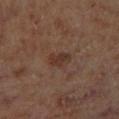Findings:
– location — the right lower leg
– subject — male, aged approximately 70
– TBP lesion metrics — an area of roughly 4 mm² and an eccentricity of roughly 0.7; roughly 7 lightness units darker than nearby skin and a lesion-to-skin contrast of about 7 (normalized; higher = more distinct); a border-irregularity rating of about 3/10, internal color variation of about 1.5 on a 0–10 scale, and a peripheral color-asymmetry measure near 0.5
– image source — ~15 mm crop, total-body skin-cancer survey
– lesion diameter — ≈2.5 mm